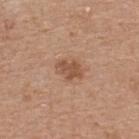biopsy status: catalogued during a skin exam; not biopsied
illumination: white-light
automated metrics: a footprint of about 5.5 mm², a shape eccentricity near 0.75, and a shape-asymmetry score of about 0.25 (0 = symmetric); an average lesion color of about L≈52 a*≈21 b*≈31 (CIELAB) and about 10 CIELAB-L* units darker than the surrounding skin; a classifier nevus-likeness of about 45/100
image: total-body-photography crop, ~15 mm field of view
subject: male, about 65 years old
diameter: ~3 mm (longest diameter)
anatomic site: the upper back